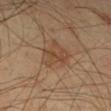follow-up = no biopsy performed (imaged during a skin exam); subject = male, about 70 years old; imaging modality = total-body-photography crop, ~15 mm field of view; illumination = cross-polarized illumination; anatomic site = the right lower leg; image-analysis metrics = a border-irregularity rating of about 4/10, a within-lesion color-variation index near 4/10, and radial color variation of about 1.5.